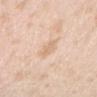Imaged during a routine full-body skin examination; the lesion was not biopsied and no histopathology is available.
A female subject in their mid-20s.
Cropped from a whole-body photographic skin survey; the tile spans about 15 mm.
About 3.5 mm across.
Captured under white-light illumination.
The lesion is located on the head or neck.